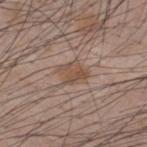  biopsy_status: not biopsied; imaged during a skin examination
  patient:
    sex: male
    age_approx: 35
  site: upper back
  image:
    source: total-body photography crop
    field_of_view_mm: 15